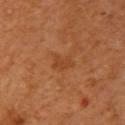lighting=cross-polarized | body site=the arm | patient=female, in their mid- to late 50s | image source=~15 mm tile from a whole-body skin photo | size=~2.5 mm (longest diameter).